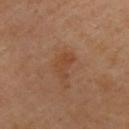{
  "biopsy_status": "not biopsied; imaged during a skin examination",
  "site": "upper back",
  "image": {
    "source": "total-body photography crop",
    "field_of_view_mm": 15
  },
  "lighting": "cross-polarized",
  "patient": {
    "sex": "male",
    "age_approx": 40
  },
  "lesion_size": {
    "long_diameter_mm_approx": 3.0
  },
  "automated_metrics": {
    "area_mm2_approx": 5.0,
    "eccentricity": 0.85,
    "shape_asymmetry": 0.35,
    "cielab_L": 44,
    "cielab_a": 21,
    "cielab_b": 33,
    "vs_skin_darker_L": 6.0,
    "vs_skin_contrast_norm": 5.5,
    "color_variation_0_10": 2.0,
    "peripheral_color_asymmetry": 0.5,
    "nevus_likeness_0_100": 5,
    "lesion_detection_confidence_0_100": 100
  }
}